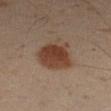Assessment: The lesion was tiled from a total-body skin photograph and was not biopsied. Context: Imaged with cross-polarized lighting. Located on the left forearm. The lesion's longest dimension is about 5.5 mm. A 15 mm crop from a total-body photograph taken for skin-cancer surveillance. A male subject, roughly 50 years of age.